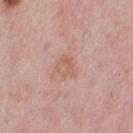| feature | finding |
|---|---|
| workup | total-body-photography surveillance lesion; no biopsy |
| acquisition | 15 mm crop, total-body photography |
| subject | female, roughly 50 years of age |
| size | ~2.5 mm (longest diameter) |
| automated lesion analysis | a classifier nevus-likeness of about 0/100 and a lesion-detection confidence of about 100/100 |
| body site | the left thigh |
| illumination | white-light |